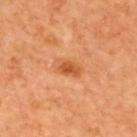Clinical impression: The lesion was tiled from a total-body skin photograph and was not biopsied. Context: A male patient approximately 65 years of age. Located on the back. The total-body-photography lesion software estimated a lesion area of about 5 mm², a shape eccentricity near 0.85, and a symmetry-axis asymmetry near 0.25. The analysis additionally found a border-irregularity index near 2/10, a within-lesion color-variation index near 3.5/10, and radial color variation of about 1. Cropped from a whole-body photographic skin survey; the tile spans about 15 mm.